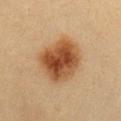Q: Was this lesion biopsied?
A: total-body-photography surveillance lesion; no biopsy
Q: Patient demographics?
A: female, about 30 years old
Q: How was this image acquired?
A: total-body-photography crop, ~15 mm field of view
Q: Lesion location?
A: the front of the torso